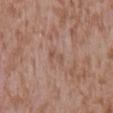biopsy_status: not biopsied; imaged during a skin examination
site: mid back
lesion_size:
  long_diameter_mm_approx: 2.5
patient:
  sex: male
  age_approx: 45
automated_metrics:
  area_mm2_approx: 2.5
  eccentricity: 0.9
  shape_asymmetry: 0.25
  cielab_L: 52
  cielab_a: 20
  cielab_b: 26
  vs_skin_darker_L: 7.0
  vs_skin_contrast_norm: 5.0
  border_irregularity_0_10: 2.5
  peripheral_color_asymmetry: 0.0
  nevus_likeness_0_100: 0
lighting: white-light
image:
  source: total-body photography crop
  field_of_view_mm: 15